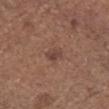Assessment: Imaged during a routine full-body skin examination; the lesion was not biopsied and no histopathology is available. Clinical summary: Cropped from a total-body skin-imaging series; the visible field is about 15 mm. The lesion is located on the head or neck. Captured under white-light illumination. About 2.5 mm across. A male patient, roughly 65 years of age. The total-body-photography lesion software estimated a footprint of about 3 mm² and a shape-asymmetry score of about 0.25 (0 = symmetric). The analysis additionally found a color-variation rating of about 2.5/10 and a peripheral color-asymmetry measure near 1.